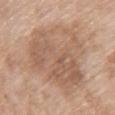Part of a total-body skin-imaging series; this lesion was reviewed on a skin check and was not flagged for biopsy.
Located on the chest.
A female subject, aged 73–77.
This is a white-light tile.
This image is a 15 mm lesion crop taken from a total-body photograph.
The recorded lesion diameter is about 9 mm.